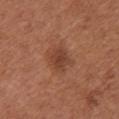biopsy_status: not biopsied; imaged during a skin examination
site: chest
patient:
  sex: female
  age_approx: 65
image:
  source: total-body photography crop
  field_of_view_mm: 15
lesion_size:
  long_diameter_mm_approx: 3.0
automated_metrics:
  area_mm2_approx: 6.5
  eccentricity: 0.55
  shape_asymmetry: 0.25
  nevus_likeness_0_100: 50
  lesion_detection_confidence_0_100: 100
lighting: white-light